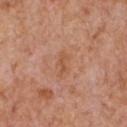The lesion was photographed on a routine skin check and not biopsied; there is no pathology result.
The lesion is on the chest.
A male subject in their mid- to late 60s.
Cropped from a whole-body photographic skin survey; the tile spans about 15 mm.